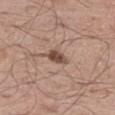follow-up = total-body-photography surveillance lesion; no biopsy | lesion diameter = about 2.5 mm | subject = male, aged 53 to 57 | automated metrics = a mean CIELAB color near L≈48 a*≈18 b*≈25 and roughly 13 lightness units darker than nearby skin; a border-irregularity rating of about 2/10, a color-variation rating of about 2.5/10, and a peripheral color-asymmetry measure near 1; a nevus-likeness score of about 90/100 and a detector confidence of about 100 out of 100 that the crop contains a lesion | imaging modality = ~15 mm tile from a whole-body skin photo | body site = the leg | tile lighting = white-light illumination.